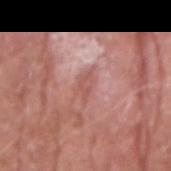| field | value |
|---|---|
| workup | total-body-photography surveillance lesion; no biopsy |
| anatomic site | the right upper arm |
| lighting | white-light |
| patient | male, approximately 65 years of age |
| image source | ~15 mm crop, total-body skin-cancer survey |